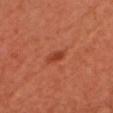This lesion was catalogued during total-body skin photography and was not selected for biopsy.
Automated tile analysis of the lesion measured a footprint of about 2.5 mm², an outline eccentricity of about 0.9 (0 = round, 1 = elongated), and two-axis asymmetry of about 0.25. And it measured a classifier nevus-likeness of about 80/100 and a detector confidence of about 100 out of 100 that the crop contains a lesion.
The tile uses cross-polarized illumination.
The recorded lesion diameter is about 2.5 mm.
A close-up tile cropped from a whole-body skin photograph, about 15 mm across.
A female patient, roughly 50 years of age.